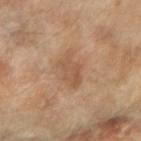A female subject, aged 68 to 72. A lesion tile, about 15 mm wide, cut from a 3D total-body photograph. Located on the arm. Imaged with cross-polarized lighting.